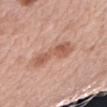Recorded during total-body skin imaging; not selected for excision or biopsy. A female subject in their mid- to late 60s. The lesion is on the right upper arm. Cropped from a total-body skin-imaging series; the visible field is about 15 mm.